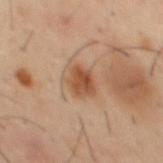Clinical impression: Imaged during a routine full-body skin examination; the lesion was not biopsied and no histopathology is available. Context: A region of skin cropped from a whole-body photographic capture, roughly 15 mm wide. Located on the mid back. The patient is a male approximately 40 years of age. The recorded lesion diameter is about 3.5 mm. This is a cross-polarized tile.